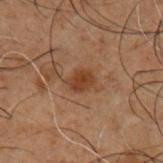biopsy_status: not biopsied; imaged during a skin examination
site: chest
patient:
  sex: male
  age_approx: 50
automated_metrics:
  area_mm2_approx: 4.5
  eccentricity: 0.7
  shape_asymmetry: 0.25
  nevus_likeness_0_100: 60
lighting: cross-polarized
image:
  source: total-body photography crop
  field_of_view_mm: 15
lesion_size:
  long_diameter_mm_approx: 2.5